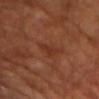Imaged during a routine full-body skin examination; the lesion was not biopsied and no histopathology is available. The lesion is on the left upper arm. The patient is a male aged 68–72. A lesion tile, about 15 mm wide, cut from a 3D total-body photograph.